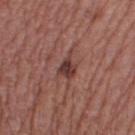Assessment: This lesion was catalogued during total-body skin photography and was not selected for biopsy. Clinical summary: On the right thigh. Automated image analysis of the tile measured a within-lesion color-variation index near 3.5/10. It also reported a nevus-likeness score of about 10/100 and a detector confidence of about 100 out of 100 that the crop contains a lesion. A female patient, aged 58–62. About 3.5 mm across. The tile uses white-light illumination. A roughly 15 mm field-of-view crop from a total-body skin photograph.